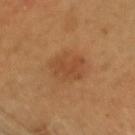No biopsy was performed on this lesion — it was imaged during a full skin examination and was not determined to be concerning.
Imaged with cross-polarized lighting.
Located on the head or neck.
Cropped from a whole-body photographic skin survey; the tile spans about 15 mm.
The patient is a male approximately 45 years of age.
Approximately 5 mm at its widest.